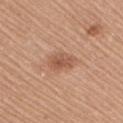Captured during whole-body skin photography for melanoma surveillance; the lesion was not biopsied. Captured under white-light illumination. About 3.5 mm across. The subject is a female about 65 years old. A roughly 15 mm field-of-view crop from a total-body skin photograph. The lesion-visualizer software estimated a color-variation rating of about 3.5/10 and radial color variation of about 1. The software also gave a nevus-likeness score of about 90/100 and a detector confidence of about 100 out of 100 that the crop contains a lesion. The lesion is located on the right upper arm.